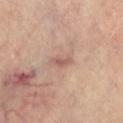This lesion was catalogued during total-body skin photography and was not selected for biopsy. Approximately 3 mm at its widest. The total-body-photography lesion software estimated an area of roughly 3.5 mm², a shape eccentricity near 0.9, and two-axis asymmetry of about 0.4. The software also gave a color-variation rating of about 1/10 and a peripheral color-asymmetry measure near 0.5. The analysis additionally found lesion-presence confidence of about 100/100. The subject is a female in their mid- to late 60s. Captured under cross-polarized illumination. From the leg. A region of skin cropped from a whole-body photographic capture, roughly 15 mm wide.